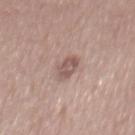{
  "biopsy_status": "not biopsied; imaged during a skin examination",
  "automated_metrics": {
    "eccentricity": 0.8,
    "shape_asymmetry": 0.4,
    "lesion_detection_confidence_0_100": 100
  },
  "site": "back",
  "lighting": "white-light",
  "patient": {
    "sex": "male",
    "age_approx": 60
  },
  "lesion_size": {
    "long_diameter_mm_approx": 3.5
  },
  "image": {
    "source": "total-body photography crop",
    "field_of_view_mm": 15
  }
}